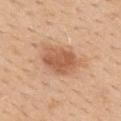Captured under white-light illumination. The subject is a female roughly 45 years of age. A close-up tile cropped from a whole-body skin photograph, about 15 mm across. Located on the mid back.